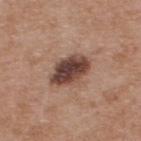Case summary:
• follow-up — imaged on a skin check; not biopsied
• location — the back
• acquisition — ~15 mm tile from a whole-body skin photo
• illumination — white-light illumination
• patient — male, in their mid- to late 50s